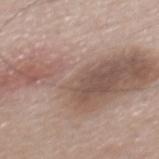Case summary:
• biopsy status — imaged on a skin check; not biopsied
• illumination — white-light illumination
• image — ~15 mm tile from a whole-body skin photo
• subject — male, aged 53–57
• site — the mid back